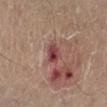The lesion was tiled from a total-body skin photograph and was not biopsied. Cropped from a whole-body photographic skin survey; the tile spans about 15 mm. On the right lower leg. An algorithmic analysis of the crop reported an eccentricity of roughly 0.5 and a shape-asymmetry score of about 0.2 (0 = symmetric). The analysis additionally found about 12 CIELAB-L* units darker than the surrounding skin and a normalized lesion–skin contrast near 9.5. It also reported a within-lesion color-variation index near 7/10. It also reported a detector confidence of about 100 out of 100 that the crop contains a lesion. A male subject about 65 years old. Captured under white-light illumination. About 2.5 mm across.